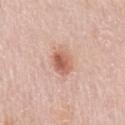Impression: Recorded during total-body skin imaging; not selected for excision or biopsy. Context: The lesion is on the left upper arm. The tile uses white-light illumination. Automated image analysis of the tile measured an average lesion color of about L≈60 a*≈25 b*≈30 (CIELAB), about 12 CIELAB-L* units darker than the surrounding skin, and a lesion-to-skin contrast of about 8 (normalized; higher = more distinct). Measured at roughly 3 mm in maximum diameter. A 15 mm close-up extracted from a 3D total-body photography capture. The subject is a female about 65 years old.